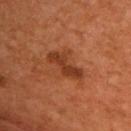Imaged during a routine full-body skin examination; the lesion was not biopsied and no histopathology is available. A male patient, aged 53–57. Approximately 5 mm at its widest. Automated tile analysis of the lesion measured an area of roughly 7.5 mm², an eccentricity of roughly 0.9, and a shape-asymmetry score of about 0.4 (0 = symmetric). It also reported a lesion color around L≈35 a*≈25 b*≈33 in CIELAB and a lesion-to-skin contrast of about 8 (normalized; higher = more distinct). The software also gave an automated nevus-likeness rating near 10 out of 100 and a lesion-detection confidence of about 100/100. From the chest. Cropped from a whole-body photographic skin survey; the tile spans about 15 mm. Captured under cross-polarized illumination.